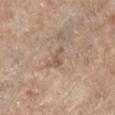notes = no biopsy performed (imaged during a skin exam) | lighting = white-light illumination | image = total-body-photography crop, ~15 mm field of view | lesion size = about 2.5 mm | site = the leg | patient = female, in their mid- to late 80s or older.